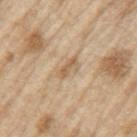biopsy_status: not biopsied; imaged during a skin examination
patient:
  sex: male
  age_approx: 70
automated_metrics:
  cielab_L: 61
  cielab_a: 15
  cielab_b: 34
  vs_skin_darker_L: 9.0
  vs_skin_contrast_norm: 6.0
  nevus_likeness_0_100: 0
  lesion_detection_confidence_0_100: 75
site: left upper arm
image:
  source: total-body photography crop
  field_of_view_mm: 15
lighting: white-light
lesion_size:
  long_diameter_mm_approx: 3.0